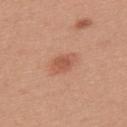Notes:
- follow-up — total-body-photography surveillance lesion; no biopsy
- imaging modality — total-body-photography crop, ~15 mm field of view
- patient — female, about 35 years old
- illumination — white-light illumination
- size — about 3.5 mm
- location — the upper back